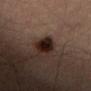Q: Was a biopsy performed?
A: total-body-photography surveillance lesion; no biopsy
Q: What is the anatomic site?
A: the right thigh
Q: Lesion size?
A: ≈3.5 mm
Q: Who is the patient?
A: male, about 70 years old
Q: What is the imaging modality?
A: ~15 mm tile from a whole-body skin photo
Q: What lighting was used for the tile?
A: cross-polarized